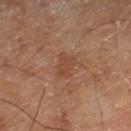| feature | finding |
|---|---|
| biopsy status | no biopsy performed (imaged during a skin exam) |
| anatomic site | the right lower leg |
| lighting | cross-polarized illumination |
| image | ~15 mm crop, total-body skin-cancer survey |
| automated metrics | a lesion color around L≈46 a*≈22 b*≈31 in CIELAB, a lesion–skin lightness drop of about 6, and a normalized border contrast of about 5.5; a border-irregularity index near 3.5/10, internal color variation of about 2.5 on a 0–10 scale, and peripheral color asymmetry of about 1 |
| subject | male, aged around 65 |